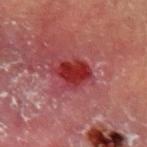Clinical impression:
The lesion was photographed on a routine skin check and not biopsied; there is no pathology result.
Background:
The lesion is on the left upper arm. This is a cross-polarized tile. Measured at roughly 3.5 mm in maximum diameter. A 15 mm close-up extracted from a 3D total-body photography capture. A male subject aged 53 to 57. The lesion-visualizer software estimated a lesion color around L≈31 a*≈36 b*≈25 in CIELAB and a normalized border contrast of about 11.5. The software also gave an automated nevus-likeness rating near 20 out of 100 and a lesion-detection confidence of about 100/100.